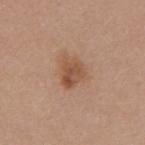Impression: Captured during whole-body skin photography for melanoma surveillance; the lesion was not biopsied. Clinical summary: The subject is a female aged 28–32. A 15 mm crop from a total-body photograph taken for skin-cancer surveillance. Located on the right upper arm. The recorded lesion diameter is about 3.5 mm. The total-body-photography lesion software estimated a lesion color around L≈52 a*≈21 b*≈31 in CIELAB, a lesion–skin lightness drop of about 10, and a normalized border contrast of about 7. The software also gave border irregularity of about 3 on a 0–10 scale, internal color variation of about 5 on a 0–10 scale, and radial color variation of about 1.5. The software also gave a lesion-detection confidence of about 100/100.